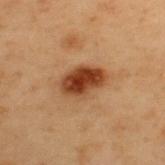follow-up = total-body-photography surveillance lesion; no biopsy | lighting = cross-polarized illumination | image source = total-body-photography crop, ~15 mm field of view | site = the upper back | patient = male, roughly 55 years of age.